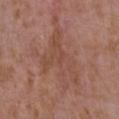Assessment:
No biopsy was performed on this lesion — it was imaged during a full skin examination and was not determined to be concerning.
Acquisition and patient details:
The lesion is on the left upper arm. A lesion tile, about 15 mm wide, cut from a 3D total-body photograph. The tile uses white-light illumination. Automated tile analysis of the lesion measured a mean CIELAB color near L≈48 a*≈21 b*≈27, roughly 6 lightness units darker than nearby skin, and a normalized lesion–skin contrast near 5. The software also gave a color-variation rating of about 3.5/10 and peripheral color asymmetry of about 1.5. The analysis additionally found lesion-presence confidence of about 60/100. A female patient, aged approximately 35. Measured at roughly 9.5 mm in maximum diameter.